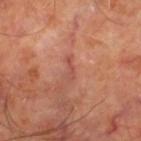Imaged during a routine full-body skin examination; the lesion was not biopsied and no histopathology is available. A subject aged 63–67. The recorded lesion diameter is about 2.5 mm. The total-body-photography lesion software estimated a footprint of about 1.5 mm² and two-axis asymmetry of about 0.65. It also reported a nevus-likeness score of about 0/100 and a lesion-detection confidence of about 95/100. The lesion is on the left thigh. This is a cross-polarized tile. This image is a 15 mm lesion crop taken from a total-body photograph.